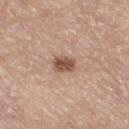No biopsy was performed on this lesion — it was imaged during a full skin examination and was not determined to be concerning. From the leg. A roughly 15 mm field-of-view crop from a total-body skin photograph. The lesion's longest dimension is about 2.5 mm. A female subject, roughly 75 years of age. Imaged with white-light lighting.